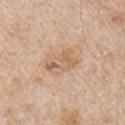<record>
  <site>right upper arm</site>
  <image>
    <source>total-body photography crop</source>
    <field_of_view_mm>15</field_of_view_mm>
  </image>
  <lesion_size>
    <long_diameter_mm_approx>4.0</long_diameter_mm_approx>
  </lesion_size>
  <patient>
    <sex>male</sex>
    <age_approx>65</age_approx>
  </patient>
  <lighting>white-light</lighting>
</record>